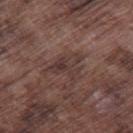Findings:
• workup · no biopsy performed (imaged during a skin exam)
• patient · male, aged around 75
• location · the right thigh
• image source · total-body-photography crop, ~15 mm field of view